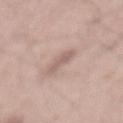No biopsy was performed on this lesion — it was imaged during a full skin examination and was not determined to be concerning. The subject is a male aged 53 to 57. A 15 mm close-up tile from a total-body photography series done for melanoma screening. The lesion is on the back. The tile uses white-light illumination. Automated image analysis of the tile measured a footprint of about 4 mm², an eccentricity of roughly 0.9, and a shape-asymmetry score of about 0.3 (0 = symmetric). The analysis additionally found a mean CIELAB color near L≈60 a*≈17 b*≈22, about 9 CIELAB-L* units darker than the surrounding skin, and a lesion-to-skin contrast of about 5.5 (normalized; higher = more distinct). And it measured a nevus-likeness score of about 0/100 and lesion-presence confidence of about 95/100.